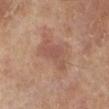Captured under cross-polarized illumination.
The lesion is located on the left lower leg.
The total-body-photography lesion software estimated a normalized border contrast of about 5.5. It also reported a border-irregularity index near 5/10, a within-lesion color-variation index near 4/10, and peripheral color asymmetry of about 1.5.
Approximately 5 mm at its widest.
A close-up tile cropped from a whole-body skin photograph, about 15 mm across.
The patient is a female approximately 75 years of age.